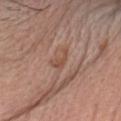Assessment: Captured during whole-body skin photography for melanoma surveillance; the lesion was not biopsied. Image and clinical context: Approximately 3 mm at its widest. Automated tile analysis of the lesion measured a border-irregularity index near 6/10, internal color variation of about 0.5 on a 0–10 scale, and peripheral color asymmetry of about 0. The lesion is located on the head or neck. A male subject aged around 80. Cropped from a whole-body photographic skin survey; the tile spans about 15 mm.